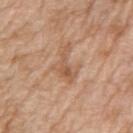No biopsy was performed on this lesion — it was imaged during a full skin examination and was not determined to be concerning. The lesion is located on the left upper arm. The subject is a male about 70 years old. A lesion tile, about 15 mm wide, cut from a 3D total-body photograph.